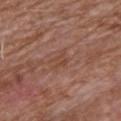image: 15 mm crop, total-body photography
patient: male, aged 58–62
site: the upper back